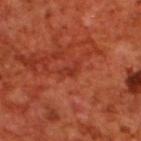Q: Was a biopsy performed?
A: imaged on a skin check; not biopsied
Q: Who is the patient?
A: male, roughly 70 years of age
Q: Where on the body is the lesion?
A: the upper back
Q: How was this image acquired?
A: ~15 mm tile from a whole-body skin photo
Q: How large is the lesion?
A: ≈2.5 mm
Q: How was the tile lit?
A: cross-polarized